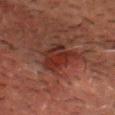A male patient, about 50 years old.
A close-up tile cropped from a whole-body skin photograph, about 15 mm across.
An algorithmic analysis of the crop reported an area of roughly 11 mm², a shape eccentricity near 0.75, and a symmetry-axis asymmetry near 0.3. And it measured a mean CIELAB color near L≈22 a*≈20 b*≈21, about 7 CIELAB-L* units darker than the surrounding skin, and a lesion-to-skin contrast of about 7.5 (normalized; higher = more distinct). It also reported a color-variation rating of about 5/10.
The tile uses cross-polarized illumination.
The lesion is on the head or neck.
About 4.5 mm across.